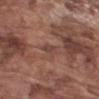{
  "biopsy_status": "not biopsied; imaged during a skin examination",
  "image": {
    "source": "total-body photography crop",
    "field_of_view_mm": 15
  },
  "lesion_size": {
    "long_diameter_mm_approx": 2.5
  },
  "lighting": "white-light",
  "site": "right upper arm",
  "patient": {
    "sex": "male",
    "age_approx": 75
  }
}